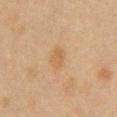Q: What are the patient's age and sex?
A: female, roughly 40 years of age
Q: Lesion location?
A: the chest
Q: How was the tile lit?
A: cross-polarized illumination
Q: How large is the lesion?
A: about 2.5 mm
Q: What did automated image analysis measure?
A: an area of roughly 4 mm², an outline eccentricity of about 0.7 (0 = round, 1 = elongated), and a symmetry-axis asymmetry near 0.2; a border-irregularity rating of about 2/10, a color-variation rating of about 1.5/10, and a peripheral color-asymmetry measure near 0.5
Q: How was this image acquired?
A: ~15 mm tile from a whole-body skin photo